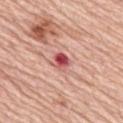Part of a total-body skin-imaging series; this lesion was reviewed on a skin check and was not flagged for biopsy. A 15 mm crop from a total-body photograph taken for skin-cancer surveillance. From the upper back. The subject is a female about 65 years old.